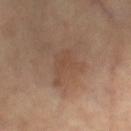notes=total-body-photography surveillance lesion; no biopsy | anatomic site=the right thigh | patient=female, aged 63 to 67 | lesion size=≈4 mm | image source=~15 mm tile from a whole-body skin photo.